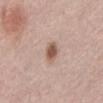{
  "biopsy_status": "not biopsied; imaged during a skin examination",
  "lesion_size": {
    "long_diameter_mm_approx": 2.5
  },
  "lighting": "white-light",
  "automated_metrics": {
    "cielab_L": 55,
    "cielab_a": 20,
    "cielab_b": 27,
    "vs_skin_darker_L": 14.0,
    "vs_skin_contrast_norm": 9.5
  },
  "image": {
    "source": "total-body photography crop",
    "field_of_view_mm": 15
  },
  "patient": {
    "sex": "female",
    "age_approx": 65
  },
  "site": "abdomen"
}